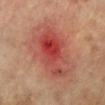The lesion was tiled from a total-body skin photograph and was not biopsied.
On the right leg.
A lesion tile, about 15 mm wide, cut from a 3D total-body photograph.
The patient is a female roughly 65 years of age.
Captured under cross-polarized illumination.
About 9 mm across.